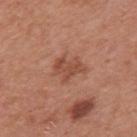Part of a total-body skin-imaging series; this lesion was reviewed on a skin check and was not flagged for biopsy. The lesion is on the back. Cropped from a whole-body photographic skin survey; the tile spans about 15 mm. A male subject, aged around 70.